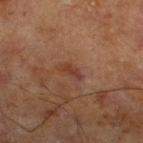{"image": {"source": "total-body photography crop", "field_of_view_mm": 15}, "patient": {"sex": "male", "age_approx": 70}, "lesion_size": {"long_diameter_mm_approx": 2.5}, "site": "left lower leg", "lighting": "cross-polarized"}